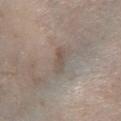Part of a total-body skin-imaging series; this lesion was reviewed on a skin check and was not flagged for biopsy. A male subject, aged 58–62. This is a white-light tile. From the left lower leg. A lesion tile, about 15 mm wide, cut from a 3D total-body photograph.